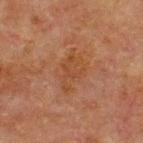| field | value |
|---|---|
| biopsy status | catalogued during a skin exam; not biopsied |
| location | the chest |
| diameter | ≈4.5 mm |
| imaging modality | total-body-photography crop, ~15 mm field of view |
| subject | male, about 65 years old |